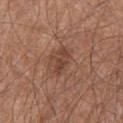Automated image analysis of the tile measured an eccentricity of roughly 0.9 and a symmetry-axis asymmetry near 0.35. It also reported an average lesion color of about L≈42 a*≈21 b*≈27 (CIELAB) and roughly 9 lightness units darker than nearby skin. The analysis additionally found a border-irregularity index near 5.5/10 and a within-lesion color-variation index near 0.5/10. On the chest. About 3.5 mm across. A lesion tile, about 15 mm wide, cut from a 3D total-body photograph. The patient is a male in their mid- to late 60s.